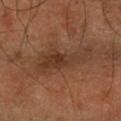This image is a 15 mm lesion crop taken from a total-body photograph.
On the arm.
The subject is a male aged around 60.
Automated image analysis of the tile measured a footprint of about 13 mm² and an outline eccentricity of about 0.95 (0 = round, 1 = elongated). The software also gave a mean CIELAB color near L≈33 a*≈19 b*≈27, about 7 CIELAB-L* units darker than the surrounding skin, and a normalized lesion–skin contrast near 7. The analysis additionally found border irregularity of about 6.5 on a 0–10 scale, a color-variation rating of about 4/10, and radial color variation of about 1.
The recorded lesion diameter is about 7.5 mm.